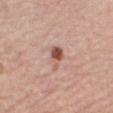The lesion was photographed on a routine skin check and not biopsied; there is no pathology result. The lesion is located on the right thigh. The total-body-photography lesion software estimated an area of roughly 4.5 mm², a shape eccentricity near 0.7, and a symmetry-axis asymmetry near 0.45. The analysis additionally found an average lesion color of about L≈54 a*≈22 b*≈27 (CIELAB) and about 13 CIELAB-L* units darker than the surrounding skin. And it measured a border-irregularity index near 4/10, a color-variation rating of about 4.5/10, and a peripheral color-asymmetry measure near 1.5. The tile uses white-light illumination. The subject is a female aged 63–67. A lesion tile, about 15 mm wide, cut from a 3D total-body photograph.